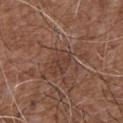The lesion is located on the chest.
Imaged with white-light lighting.
About 3 mm across.
A male patient about 75 years old.
A close-up tile cropped from a whole-body skin photograph, about 15 mm across.